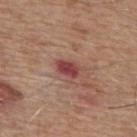Q: Was this lesion biopsied?
A: total-body-photography surveillance lesion; no biopsy
Q: What lighting was used for the tile?
A: white-light
Q: What kind of image is this?
A: 15 mm crop, total-body photography
Q: Where on the body is the lesion?
A: the mid back
Q: What is the lesion's diameter?
A: ≈3 mm
Q: What are the patient's age and sex?
A: male, aged 63 to 67